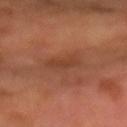follow-up: catalogued during a skin exam; not biopsied
lighting: cross-polarized
automated lesion analysis: an area of roughly 6 mm² and an outline eccentricity of about 0.8 (0 = round, 1 = elongated); a border-irregularity rating of about 2.5/10 and a peripheral color-asymmetry measure near 0.5; an automated nevus-likeness rating near 0 out of 100 and lesion-presence confidence of about 100/100
lesion diameter: about 3.5 mm
subject: male, roughly 65 years of age
image source: total-body-photography crop, ~15 mm field of view